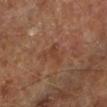Clinical impression: The lesion was tiled from a total-body skin photograph and was not biopsied. Image and clinical context: The total-body-photography lesion software estimated a normalized border contrast of about 5.5. It also reported an automated nevus-likeness rating near 0 out of 100 and lesion-presence confidence of about 100/100. The lesion is located on the left lower leg. This is a cross-polarized tile. Approximately 3 mm at its widest. A patient roughly 65 years of age. A lesion tile, about 15 mm wide, cut from a 3D total-body photograph.